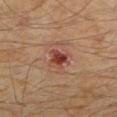The lesion was tiled from a total-body skin photograph and was not biopsied. The recorded lesion diameter is about 2.5 mm. A lesion tile, about 15 mm wide, cut from a 3D total-body photograph. The lesion is located on the left lower leg. A male subject, roughly 55 years of age.